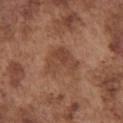  biopsy_status: not biopsied; imaged during a skin examination
  lighting: white-light
  image:
    source: total-body photography crop
    field_of_view_mm: 15
  patient:
    sex: male
    age_approx: 75
  automated_metrics:
    area_mm2_approx: 10.0
    eccentricity: 0.65
    shape_asymmetry: 0.45
    cielab_L: 44
    cielab_a: 21
    cielab_b: 29
    vs_skin_darker_L: 8.0
    vs_skin_contrast_norm: 6.0
    nevus_likeness_0_100: 0
  lesion_size:
    long_diameter_mm_approx: 4.0
  site: front of the torso